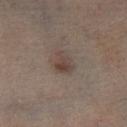Notes:
- workup · imaged on a skin check; not biopsied
- automated lesion analysis · a footprint of about 4.5 mm², an outline eccentricity of about 0.65 (0 = round, 1 = elongated), and a symmetry-axis asymmetry near 0.35; a mean CIELAB color near L≈40 a*≈14 b*≈20 and about 8 CIELAB-L* units darker than the surrounding skin; a border-irregularity index near 3.5/10, internal color variation of about 3 on a 0–10 scale, and peripheral color asymmetry of about 1
- imaging modality · ~15 mm tile from a whole-body skin photo
- lesion size · ≈2.5 mm
- illumination · cross-polarized
- subject · male, about 50 years old
- anatomic site · the left leg